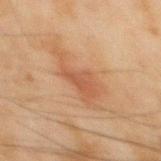biopsy status: catalogued during a skin exam; not biopsied | imaging modality: 15 mm crop, total-body photography | body site: the mid back | subject: male, in their mid-40s.